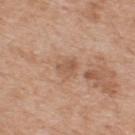Clinical summary:
The lesion-visualizer software estimated a border-irregularity index near 3/10, internal color variation of about 2.5 on a 0–10 scale, and radial color variation of about 1. The analysis additionally found a classifier nevus-likeness of about 0/100 and a lesion-detection confidence of about 100/100. A 15 mm crop from a total-body photograph taken for skin-cancer surveillance. Imaged with white-light lighting. About 2.5 mm across. The lesion is on the upper back. A male subject about 55 years old.